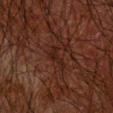Measured at roughly 3 mm in maximum diameter. From the right forearm. Captured under cross-polarized illumination. A male subject approximately 60 years of age. A 15 mm close-up extracted from a 3D total-body photography capture.